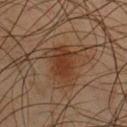biopsy status = no biopsy performed (imaged during a skin exam)
subject = male, roughly 45 years of age
acquisition = total-body-photography crop, ~15 mm field of view
body site = the chest